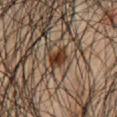Imaged during a routine full-body skin examination; the lesion was not biopsied and no histopathology is available. A lesion tile, about 15 mm wide, cut from a 3D total-body photograph. The lesion-visualizer software estimated an area of roughly 4.5 mm² and a shape-asymmetry score of about 0.25 (0 = symmetric). The analysis additionally found a mean CIELAB color near L≈33 a*≈18 b*≈28, roughly 13 lightness units darker than nearby skin, and a normalized border contrast of about 12. And it measured a lesion-detection confidence of about 85/100. A male subject aged around 45. The lesion is on the abdomen.